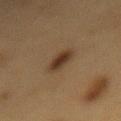Clinical impression:
Captured during whole-body skin photography for melanoma surveillance; the lesion was not biopsied.
Clinical summary:
The lesion is on the mid back. Cropped from a whole-body photographic skin survey; the tile spans about 15 mm. The patient is a male in their mid- to late 80s or older.